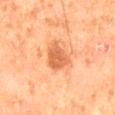Captured during whole-body skin photography for melanoma surveillance; the lesion was not biopsied. Longest diameter approximately 4 mm. The lesion is located on the mid back. A male subject, about 65 years old. This image is a 15 mm lesion crop taken from a total-body photograph.